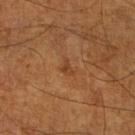Recorded during total-body skin imaging; not selected for excision or biopsy.